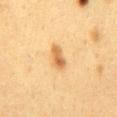Recorded during total-body skin imaging; not selected for excision or biopsy.
A female subject in their 40s.
Measured at roughly 3 mm in maximum diameter.
This is a cross-polarized tile.
On the mid back.
An algorithmic analysis of the crop reported a lesion area of about 4 mm² and an eccentricity of roughly 0.9. It also reported internal color variation of about 3 on a 0–10 scale and peripheral color asymmetry of about 1. The software also gave lesion-presence confidence of about 100/100.
A 15 mm close-up extracted from a 3D total-body photography capture.